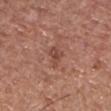The lesion was tiled from a total-body skin photograph and was not biopsied. The recorded lesion diameter is about 3 mm. A male patient, aged 63 to 67. A 15 mm crop from a total-body photograph taken for skin-cancer surveillance. The lesion is located on the chest.